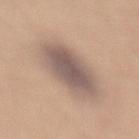Q: Was a biopsy performed?
A: no biopsy performed (imaged during a skin exam)
Q: What is the anatomic site?
A: the lower back
Q: What is the lesion's diameter?
A: ~6 mm (longest diameter)
Q: What kind of image is this?
A: ~15 mm tile from a whole-body skin photo
Q: Automated lesion metrics?
A: an area of roughly 20 mm², an outline eccentricity of about 0.75 (0 = round, 1 = elongated), and two-axis asymmetry of about 0.1; a mean CIELAB color near L≈55 a*≈14 b*≈22, about 12 CIELAB-L* units darker than the surrounding skin, and a normalized border contrast of about 9; a border-irregularity rating of about 1.5/10, a within-lesion color-variation index near 4/10, and peripheral color asymmetry of about 1; a lesion-detection confidence of about 75/100
Q: Patient demographics?
A: male, aged around 35